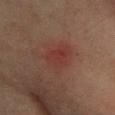{"biopsy_status": "not biopsied; imaged during a skin examination", "automated_metrics": {"cielab_L": 31, "cielab_a": 22, "cielab_b": 21, "vs_skin_darker_L": 5.0, "vs_skin_contrast_norm": 5.0, "nevus_likeness_0_100": 0}, "lesion_size": {"long_diameter_mm_approx": 4.0}, "patient": {"sex": "female", "age_approx": 55}, "site": "left lower leg", "image": {"source": "total-body photography crop", "field_of_view_mm": 15}}